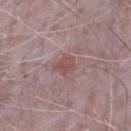Background: Automated image analysis of the tile measured an average lesion color of about L≈50 a*≈22 b*≈20 (CIELAB) and a normalized lesion–skin contrast near 6.5. And it measured border irregularity of about 3 on a 0–10 scale, a within-lesion color-variation index near 2/10, and peripheral color asymmetry of about 1. It also reported an automated nevus-likeness rating near 20 out of 100. On the chest. A 15 mm crop from a total-body photograph taken for skin-cancer surveillance. Approximately 2.5 mm at its widest. The patient is a male about 65 years old.